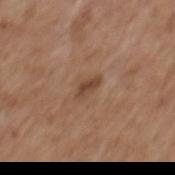Imaged during a routine full-body skin examination; the lesion was not biopsied and no histopathology is available. The tile uses white-light illumination. Measured at roughly 2.5 mm in maximum diameter. A region of skin cropped from a whole-body photographic capture, roughly 15 mm wide. A female subject, aged around 65. The lesion is on the mid back.